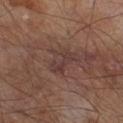Captured during whole-body skin photography for melanoma surveillance; the lesion was not biopsied. The tile uses cross-polarized illumination. A male subject about 65 years old. Approximately 3.5 mm at its widest. Cropped from a total-body skin-imaging series; the visible field is about 15 mm. The total-body-photography lesion software estimated a footprint of about 6.5 mm², an outline eccentricity of about 0.8 (0 = round, 1 = elongated), and two-axis asymmetry of about 0.25. Located on the right lower leg.